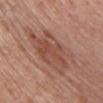This lesion was catalogued during total-body skin photography and was not selected for biopsy. The total-body-photography lesion software estimated a mean CIELAB color near L≈49 a*≈22 b*≈28, a lesion–skin lightness drop of about 9, and a lesion-to-skin contrast of about 6.5 (normalized; higher = more distinct). It also reported a border-irregularity index near 4.5/10, internal color variation of about 4 on a 0–10 scale, and peripheral color asymmetry of about 1. The tile uses white-light illumination. A female patient in their 50s. Located on the front of the torso. A roughly 15 mm field-of-view crop from a total-body skin photograph. The lesion's longest dimension is about 8 mm.